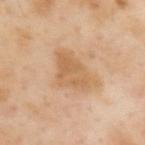biopsy status: total-body-photography surveillance lesion; no biopsy
image: ~15 mm crop, total-body skin-cancer survey
location: the back
lesion size: ~5.5 mm (longest diameter)
tile lighting: cross-polarized illumination
subject: male, aged around 55